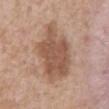Q: Was this lesion biopsied?
A: imaged on a skin check; not biopsied
Q: How was the tile lit?
A: white-light illumination
Q: Who is the patient?
A: male, aged 58 to 62
Q: Automated lesion metrics?
A: a mean CIELAB color near L≈54 a*≈19 b*≈29, roughly 11 lightness units darker than nearby skin, and a normalized lesion–skin contrast near 8; border irregularity of about 3.5 on a 0–10 scale and a peripheral color-asymmetry measure near 1; an automated nevus-likeness rating near 40 out of 100
Q: Lesion size?
A: ~7.5 mm (longest diameter)
Q: What is the imaging modality?
A: total-body-photography crop, ~15 mm field of view
Q: Lesion location?
A: the chest